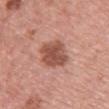* subject — female, roughly 60 years of age
* lesion size — ~4.5 mm (longest diameter)
* body site — the chest
* imaging modality — ~15 mm tile from a whole-body skin photo